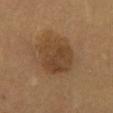Clinical impression: The lesion was photographed on a routine skin check and not biopsied; there is no pathology result. Context: From the mid back. The lesion-visualizer software estimated a mean CIELAB color near L≈40 a*≈16 b*≈31 and roughly 8 lightness units darker than nearby skin. A male subject, aged 58 to 62. The lesion's longest dimension is about 5.5 mm. A region of skin cropped from a whole-body photographic capture, roughly 15 mm wide.